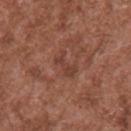The lesion was tiled from a total-body skin photograph and was not biopsied.
The total-body-photography lesion software estimated a mean CIELAB color near L≈40 a*≈23 b*≈27, roughly 6 lightness units darker than nearby skin, and a lesion-to-skin contrast of about 5.5 (normalized; higher = more distinct).
A roughly 15 mm field-of-view crop from a total-body skin photograph.
Located on the upper back.
This is a white-light tile.
Longest diameter approximately 3 mm.
The subject is a male aged 43 to 47.